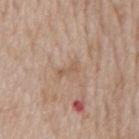| field | value |
|---|---|
| notes | no biopsy performed (imaged during a skin exam) |
| subject | male, aged 63–67 |
| automated metrics | an area of roughly 2.5 mm², a shape eccentricity near 0.9, and two-axis asymmetry of about 0.4 |
| imaging modality | total-body-photography crop, ~15 mm field of view |
| location | the back |
| illumination | white-light |